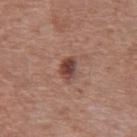Notes:
• workup — total-body-photography surveillance lesion; no biopsy
• image — 15 mm crop, total-body photography
• size — about 3 mm
• subject — male, about 75 years old
• body site — the abdomen
• lighting — white-light illumination
• automated lesion analysis — a border-irregularity index near 2/10, a color-variation rating of about 5.5/10, and peripheral color asymmetry of about 1.5